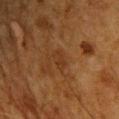The lesion was photographed on a routine skin check and not biopsied; there is no pathology result. A male subject, aged 58–62. Captured under cross-polarized illumination. On the upper back. Cropped from a total-body skin-imaging series; the visible field is about 15 mm.